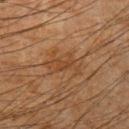<lesion>
<biopsy_status>not biopsied; imaged during a skin examination</biopsy_status>
<patient>
  <sex>male</sex>
  <age_approx>65</age_approx>
</patient>
<site>left upper arm</site>
<lesion_size>
  <long_diameter_mm_approx>4.5</long_diameter_mm_approx>
</lesion_size>
<image>
  <source>total-body photography crop</source>
  <field_of_view_mm>15</field_of_view_mm>
</image>
<lighting>cross-polarized</lighting>
</lesion>